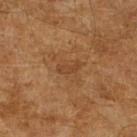biopsy_status: not biopsied; imaged during a skin examination
automated_metrics:
  area_mm2_approx: 2.5
  eccentricity: 0.9
  shape_asymmetry: 0.4
lighting: cross-polarized
patient:
  sex: male
  age_approx: 65
image:
  source: total-body photography crop
  field_of_view_mm: 15
lesion_size:
  long_diameter_mm_approx: 2.5